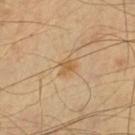Assessment: Recorded during total-body skin imaging; not selected for excision or biopsy. Image and clinical context: This is a cross-polarized tile. The lesion is on the left lower leg. A male patient approximately 65 years of age. A 15 mm close-up tile from a total-body photography series done for melanoma screening.